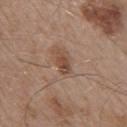Captured during whole-body skin photography for melanoma surveillance; the lesion was not biopsied.
The tile uses white-light illumination.
A roughly 15 mm field-of-view crop from a total-body skin photograph.
The recorded lesion diameter is about 3 mm.
An algorithmic analysis of the crop reported an outline eccentricity of about 0.85 (0 = round, 1 = elongated) and a symmetry-axis asymmetry near 0.3. The analysis additionally found a mean CIELAB color near L≈47 a*≈19 b*≈28, a lesion–skin lightness drop of about 10, and a normalized lesion–skin contrast near 7.5.
The lesion is located on the right upper arm.
A male patient, aged 53–57.